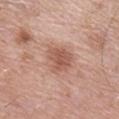The lesion was tiled from a total-body skin photograph and was not biopsied. Longest diameter approximately 4 mm. A male subject aged 78 to 82. Captured under white-light illumination. Automated image analysis of the tile measured an area of roughly 9 mm² and two-axis asymmetry of about 0.3. And it measured roughly 10 lightness units darker than nearby skin. The software also gave a border-irregularity rating of about 3/10 and internal color variation of about 2.5 on a 0–10 scale. It also reported a classifier nevus-likeness of about 65/100 and lesion-presence confidence of about 100/100. The lesion is on the leg. A close-up tile cropped from a whole-body skin photograph, about 15 mm across.